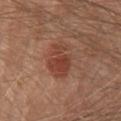| feature | finding |
|---|---|
| notes | no biopsy performed (imaged during a skin exam) |
| image | ~15 mm crop, total-body skin-cancer survey |
| lesion diameter | ~4.5 mm (longest diameter) |
| tile lighting | white-light illumination |
| subject | male, aged 78 to 82 |
| body site | the chest |